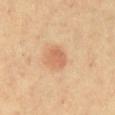The lesion was photographed on a routine skin check and not biopsied; there is no pathology result. A subject aged around 60. A roughly 15 mm field-of-view crop from a total-body skin photograph. From the front of the torso.